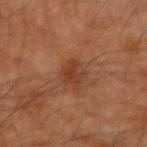Case summary:
- workup: total-body-photography surveillance lesion; no biopsy
- tile lighting: cross-polarized illumination
- diameter: ≈3 mm
- automated metrics: a border-irregularity rating of about 2.5/10 and radial color variation of about 0.5; a nevus-likeness score of about 30/100 and lesion-presence confidence of about 100/100
- subject: male, about 60 years old
- image: ~15 mm crop, total-body skin-cancer survey
- location: the right forearm